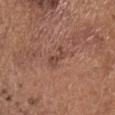Notes:
- biopsy status: catalogued during a skin exam; not biopsied
- size: ≈3.5 mm
- patient: male, aged approximately 75
- image-analysis metrics: a classifier nevus-likeness of about 10/100
- tile lighting: white-light illumination
- anatomic site: the head or neck
- image source: ~15 mm crop, total-body skin-cancer survey